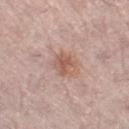Assessment: The lesion was photographed on a routine skin check and not biopsied; there is no pathology result. Acquisition and patient details: A 15 mm crop from a total-body photograph taken for skin-cancer surveillance. This is a white-light tile. A female subject, aged around 65. On the left leg.